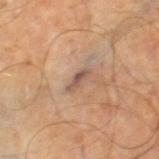follow-up: total-body-photography surveillance lesion; no biopsy.